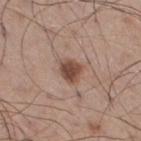patient:
  sex: male
  age_approx: 60
site: right thigh
image:
  source: total-body photography crop
  field_of_view_mm: 15
lighting: white-light
lesion_size:
  long_diameter_mm_approx: 3.0